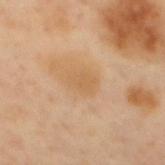No biopsy was performed on this lesion — it was imaged during a full skin examination and was not determined to be concerning. A male patient, in their 40s. A 15 mm close-up extracted from a 3D total-body photography capture. Located on the mid back.